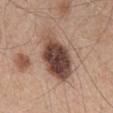| key | value |
|---|---|
| notes | imaged on a skin check; not biopsied |
| site | the mid back |
| size | ~6.5 mm (longest diameter) |
| patient | male, aged 43 to 47 |
| automated lesion analysis | a lesion area of about 21 mm² |
| image | 15 mm crop, total-body photography |